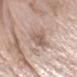| field | value |
|---|---|
| automated lesion analysis | a lesion area of about 7 mm² |
| anatomic site | the right forearm |
| image | 15 mm crop, total-body photography |
| patient | female, in their mid-70s |
| illumination | white-light |